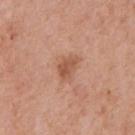Clinical impression:
Imaged during a routine full-body skin examination; the lesion was not biopsied and no histopathology is available.
Background:
The recorded lesion diameter is about 3 mm. Imaged with white-light lighting. This image is a 15 mm lesion crop taken from a total-body photograph. From the front of the torso. The total-body-photography lesion software estimated a lesion color around L≈54 a*≈24 b*≈32 in CIELAB, roughly 10 lightness units darker than nearby skin, and a normalized lesion–skin contrast near 7. It also reported a border-irregularity index near 3.5/10 and peripheral color asymmetry of about 1. And it measured a classifier nevus-likeness of about 20/100. A male patient roughly 70 years of age.